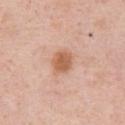Q: Was a biopsy performed?
A: catalogued during a skin exam; not biopsied
Q: How was this image acquired?
A: ~15 mm tile from a whole-body skin photo
Q: Lesion location?
A: the chest
Q: Patient demographics?
A: male, aged 48 to 52